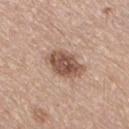Part of a total-body skin-imaging series; this lesion was reviewed on a skin check and was not flagged for biopsy.
From the right thigh.
The patient is a male aged 63–67.
This image is a 15 mm lesion crop taken from a total-body photograph.